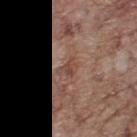Located on the upper back.
A male patient about 70 years old.
A region of skin cropped from a whole-body photographic capture, roughly 15 mm wide.
Imaged with white-light lighting.
The recorded lesion diameter is about 2.5 mm.